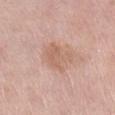<record>
  <biopsy_status>not biopsied; imaged during a skin examination</biopsy_status>
  <image>
    <source>total-body photography crop</source>
    <field_of_view_mm>15</field_of_view_mm>
  </image>
  <lighting>white-light</lighting>
  <patient>
    <sex>female</sex>
    <age_approx>40</age_approx>
  </patient>
  <site>right lower leg</site>
  <lesion_size>
    <long_diameter_mm_approx>4.0</long_diameter_mm_approx>
  </lesion_size>
</record>